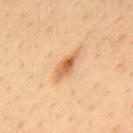  biopsy_status: not biopsied; imaged during a skin examination
  site: upper back
  lighting: cross-polarized
  patient:
    sex: male
    age_approx: 35
  lesion_size:
    long_diameter_mm_approx: 4.0
  image:
    source: total-body photography crop
    field_of_view_mm: 15